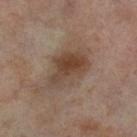workup: total-body-photography surveillance lesion; no biopsy | subject: female, about 55 years old | lighting: cross-polarized illumination | imaging modality: ~15 mm tile from a whole-body skin photo | diameter: ~6.5 mm (longest diameter) | site: the right thigh.